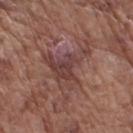biopsy status: no biopsy performed (imaged during a skin exam) | anatomic site: the right upper arm | automated metrics: a footprint of about 14 mm²; a border-irregularity rating of about 9.5/10, internal color variation of about 4.5 on a 0–10 scale, and radial color variation of about 1.5 | subject: male, aged around 75 | lighting: white-light illumination | lesion size: ≈5.5 mm | image: total-body-photography crop, ~15 mm field of view.